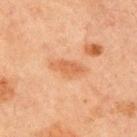Assessment:
The lesion was photographed on a routine skin check and not biopsied; there is no pathology result.
Image and clinical context:
The lesion is located on the chest. A lesion tile, about 15 mm wide, cut from a 3D total-body photograph. The lesion's longest dimension is about 4 mm. Captured under cross-polarized illumination. A male subject about 65 years old.